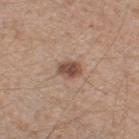<lesion>
  <biopsy_status>not biopsied; imaged during a skin examination</biopsy_status>
  <patient>
    <sex>male</sex>
    <age_approx>65</age_approx>
  </patient>
  <image>
    <source>total-body photography crop</source>
    <field_of_view_mm>15</field_of_view_mm>
  </image>
  <site>leg</site>
  <automated_metrics>
    <eccentricity>0.7</eccentricity>
    <nevus_likeness_0_100>90</nevus_likeness_0_100>
    <lesion_detection_confidence_0_100>100</lesion_detection_confidence_0_100>
  </automated_metrics>
  <lesion_size>
    <long_diameter_mm_approx>3.0</long_diameter_mm_approx>
  </lesion_size>
  <lighting>white-light</lighting>
</lesion>